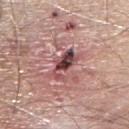Findings:
* notes — total-body-photography surveillance lesion; no biopsy
* image — 15 mm crop, total-body photography
* anatomic site — the right upper arm
* patient — male, in their mid- to late 60s